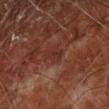| key | value |
|---|---|
| site | the left forearm |
| tile lighting | cross-polarized illumination |
| patient | in their mid-60s |
| imaging modality | ~15 mm tile from a whole-body skin photo |
| size | about 2.5 mm |